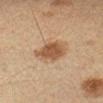lesion diameter: ~3.5 mm (longest diameter)
lighting: cross-polarized
anatomic site: the arm
acquisition: 15 mm crop, total-body photography
patient: female, aged 43–47